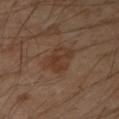  biopsy_status: not biopsied; imaged during a skin examination
  image:
    source: total-body photography crop
    field_of_view_mm: 15
  site: left forearm
  patient:
    sex: male
    age_approx: 45